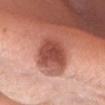{"biopsy_status": "not biopsied; imaged during a skin examination", "site": "head or neck", "image": {"source": "total-body photography crop", "field_of_view_mm": 15}, "lighting": "white-light", "patient": {"sex": "female", "age_approx": 40}}